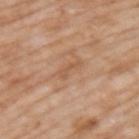Notes:
- workup: catalogued during a skin exam; not biopsied
- subject: male, about 60 years old
- acquisition: total-body-photography crop, ~15 mm field of view
- tile lighting: white-light
- anatomic site: the upper back